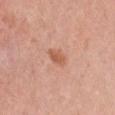Impression: Part of a total-body skin-imaging series; this lesion was reviewed on a skin check and was not flagged for biopsy. Context: A female patient approximately 35 years of age. From the chest. About 2.5 mm across. This image is a 15 mm lesion crop taken from a total-body photograph. Imaged with white-light lighting.